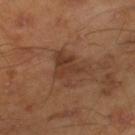Clinical impression: The lesion was photographed on a routine skin check and not biopsied; there is no pathology result. Background: This image is a 15 mm lesion crop taken from a total-body photograph. Located on the right lower leg. A male patient, aged approximately 45.